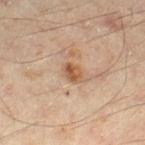Recorded during total-body skin imaging; not selected for excision or biopsy. A 15 mm close-up tile from a total-body photography series done for melanoma screening. The lesion's longest dimension is about 2.5 mm. A male subject aged 43 to 47. Located on the leg.